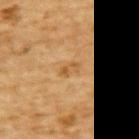Findings:
• patient — male, aged 83 or over
• tile lighting — cross-polarized illumination
• location — the upper back
• automated lesion analysis — a lesion area of about 2.5 mm² and a shape eccentricity near 0.85; roughly 7 lightness units darker than nearby skin; border irregularity of about 4.5 on a 0–10 scale, a color-variation rating of about 0/10, and peripheral color asymmetry of about 0; an automated nevus-likeness rating near 0 out of 100 and a lesion-detection confidence of about 100/100
• image source — 15 mm crop, total-body photography
• diameter — about 2.5 mm